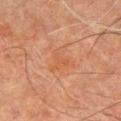– workup · catalogued during a skin exam; not biopsied
– image source · ~15 mm crop, total-body skin-cancer survey
– patient · male, aged approximately 65
– site · the chest